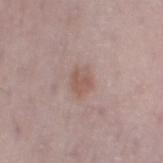  biopsy_status: not biopsied; imaged during a skin examination
  patient:
    sex: female
    age_approx: 30
  lighting: white-light
  site: left thigh
  image:
    source: total-body photography crop
    field_of_view_mm: 15